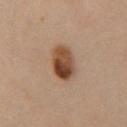Imaged during a routine full-body skin examination; the lesion was not biopsied and no histopathology is available.
Located on the chest.
The subject is a male aged 63–67.
A region of skin cropped from a whole-body photographic capture, roughly 15 mm wide.